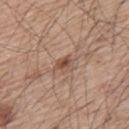<record>
<biopsy_status>not biopsied; imaged during a skin examination</biopsy_status>
<site>back</site>
<image>
  <source>total-body photography crop</source>
  <field_of_view_mm>15</field_of_view_mm>
</image>
<lighting>white-light</lighting>
<patient>
  <sex>male</sex>
  <age_approx>65</age_approx>
</patient>
<lesion_size>
  <long_diameter_mm_approx>2.5</long_diameter_mm_approx>
</lesion_size>
</record>